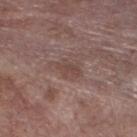| key | value |
|---|---|
| workup | no biopsy performed (imaged during a skin exam) |
| illumination | white-light illumination |
| patient | male, roughly 55 years of age |
| lesion diameter | ~3.5 mm (longest diameter) |
| TBP lesion metrics | a mean CIELAB color near L≈45 a*≈17 b*≈21 and a lesion-to-skin contrast of about 5.5 (normalized; higher = more distinct); a border-irregularity rating of about 4.5/10 and a within-lesion color-variation index near 1.5/10 |
| acquisition | ~15 mm crop, total-body skin-cancer survey |
| site | the left lower leg |